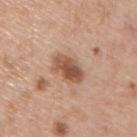- follow-up: no biopsy performed (imaged during a skin exam)
- image source: 15 mm crop, total-body photography
- location: the upper back
- image-analysis metrics: border irregularity of about 2.5 on a 0–10 scale, internal color variation of about 5.5 on a 0–10 scale, and peripheral color asymmetry of about 2; a lesion-detection confidence of about 100/100
- patient: male, aged 38 to 42
- lighting: white-light
- diameter: ≈4.5 mm